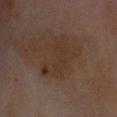Impression: Part of a total-body skin-imaging series; this lesion was reviewed on a skin check and was not flagged for biopsy. Clinical summary: A 15 mm close-up tile from a total-body photography series done for melanoma screening. Imaged with cross-polarized lighting. Longest diameter approximately 6.5 mm. A male subject aged approximately 70. On the head or neck.